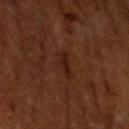Imaged during a routine full-body skin examination; the lesion was not biopsied and no histopathology is available.